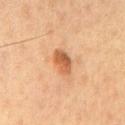Findings:
• follow-up: no biopsy performed (imaged during a skin exam)
• image: total-body-photography crop, ~15 mm field of view
• anatomic site: the front of the torso
• diameter: ~3.5 mm (longest diameter)
• patient: male, roughly 60 years of age